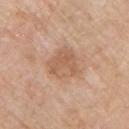Assessment:
The lesion was photographed on a routine skin check and not biopsied; there is no pathology result.
Background:
A male patient aged approximately 75. Imaged with white-light lighting. A region of skin cropped from a whole-body photographic capture, roughly 15 mm wide. Located on the arm.